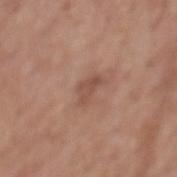No biopsy was performed on this lesion — it was imaged during a full skin examination and was not determined to be concerning.
From the mid back.
A close-up tile cropped from a whole-body skin photograph, about 15 mm across.
Automated image analysis of the tile measured a border-irregularity rating of about 4.5/10, a within-lesion color-variation index near 1.5/10, and radial color variation of about 0.5.
A male patient aged 73–77.
The tile uses white-light illumination.